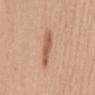No biopsy was performed on this lesion — it was imaged during a full skin examination and was not determined to be concerning. A 15 mm crop from a total-body photograph taken for skin-cancer surveillance. A female patient, aged around 50. Automated tile analysis of the lesion measured a border-irregularity index near 3/10. And it measured a nevus-likeness score of about 75/100. About 4.5 mm across. On the mid back. Imaged with white-light lighting.